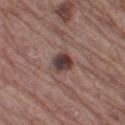{"biopsy_status": "not biopsied; imaged during a skin examination", "site": "left thigh", "lighting": "white-light", "patient": {"sex": "male", "age_approx": 65}, "automated_metrics": {"border_irregularity_0_10": 2.0, "color_variation_0_10": 6.5}, "image": {"source": "total-body photography crop", "field_of_view_mm": 15}}